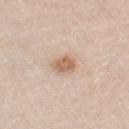Imaged during a routine full-body skin examination; the lesion was not biopsied and no histopathology is available. The total-body-photography lesion software estimated an area of roughly 5 mm², an outline eccentricity of about 0.65 (0 = round, 1 = elongated), and two-axis asymmetry of about 0.25. And it measured an automated nevus-likeness rating near 85 out of 100 and lesion-presence confidence of about 100/100. Located on the chest. A male subject, approximately 40 years of age. A lesion tile, about 15 mm wide, cut from a 3D total-body photograph.